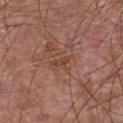image:
  source: total-body photography crop
  field_of_view_mm: 15
patient:
  sex: male
  age_approx: 65
site: chest